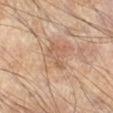Q: What is the imaging modality?
A: ~15 mm tile from a whole-body skin photo
Q: Automated lesion metrics?
A: a border-irregularity rating of about 6.5/10, a color-variation rating of about 0/10, and peripheral color asymmetry of about 0
Q: Lesion size?
A: ≈3.5 mm
Q: Patient demographics?
A: male, roughly 65 years of age
Q: How was the tile lit?
A: cross-polarized
Q: Where on the body is the lesion?
A: the right lower leg